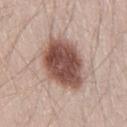Impression: Part of a total-body skin-imaging series; this lesion was reviewed on a skin check and was not flagged for biopsy. Context: A male subject, roughly 40 years of age. Measured at roughly 7 mm in maximum diameter. A lesion tile, about 15 mm wide, cut from a 3D total-body photograph. The tile uses white-light illumination. The lesion is located on the right thigh.